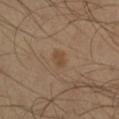This lesion was catalogued during total-body skin photography and was not selected for biopsy.
A male patient, roughly 30 years of age.
The lesion's longest dimension is about 2 mm.
Imaged with cross-polarized lighting.
The lesion is on the leg.
A 15 mm close-up tile from a total-body photography series done for melanoma screening.
Automated image analysis of the tile measured a footprint of about 2.5 mm², an outline eccentricity of about 0.75 (0 = round, 1 = elongated), and two-axis asymmetry of about 0.2. It also reported a nevus-likeness score of about 35/100 and a lesion-detection confidence of about 100/100.